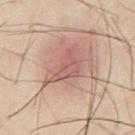workup: total-body-photography surveillance lesion; no biopsy | acquisition: total-body-photography crop, ~15 mm field of view | subject: male, in their mid- to late 50s | TBP lesion metrics: a color-variation rating of about 2/10 | illumination: cross-polarized illumination | site: the abdomen.